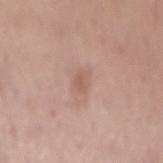<case>
  <biopsy_status>not biopsied; imaged during a skin examination</biopsy_status>
  <site>mid back</site>
  <lesion_size>
    <long_diameter_mm_approx>2.5</long_diameter_mm_approx>
  </lesion_size>
  <patient>
    <sex>female</sex>
    <age_approx>65</age_approx>
  </patient>
  <image>
    <source>total-body photography crop</source>
    <field_of_view_mm>15</field_of_view_mm>
  </image>
  <automated_metrics>
    <area_mm2_approx>3.5</area_mm2_approx>
    <eccentricity>0.85</eccentricity>
    <cielab_L>58</cielab_L>
    <cielab_a>19</cielab_a>
    <cielab_b>26</cielab_b>
    <vs_skin_darker_L>7.0</vs_skin_darker_L>
    <vs_skin_contrast_norm>5.0</vs_skin_contrast_norm>
    <nevus_likeness_0_100>0</nevus_likeness_0_100>
    <lesion_detection_confidence_0_100>100</lesion_detection_confidence_0_100>
  </automated_metrics>
  <lighting>white-light</lighting>
</case>